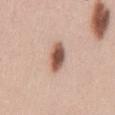| key | value |
|---|---|
| notes | imaged on a skin check; not biopsied |
| image source | 15 mm crop, total-body photography |
| lighting | white-light |
| diameter | ≈4 mm |
| anatomic site | the abdomen |
| image-analysis metrics | an area of roughly 6.5 mm²; an average lesion color of about L≈54 a*≈21 b*≈28 (CIELAB) and a normalized border contrast of about 11.5; border irregularity of about 2.5 on a 0–10 scale; a classifier nevus-likeness of about 100/100 |
| subject | male, aged around 45 |